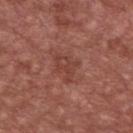Captured during whole-body skin photography for melanoma surveillance; the lesion was not biopsied. The lesion is located on the chest. This is a white-light tile. The patient is a male in their mid-60s. Automated tile analysis of the lesion measured a lesion area of about 5.5 mm² and an eccentricity of roughly 0.7. Cropped from a whole-body photographic skin survey; the tile spans about 15 mm. Longest diameter approximately 3.5 mm.